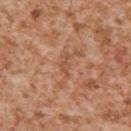The lesion was photographed on a routine skin check and not biopsied; there is no pathology result.
Cropped from a whole-body photographic skin survey; the tile spans about 15 mm.
A male subject in their mid- to late 40s.
The lesion is located on the right upper arm.